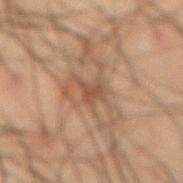Located on the front of the torso. A male subject roughly 50 years of age. A lesion tile, about 15 mm wide, cut from a 3D total-body photograph.